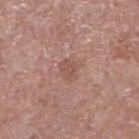{
  "biopsy_status": "not biopsied; imaged during a skin examination",
  "image": {
    "source": "total-body photography crop",
    "field_of_view_mm": 15
  },
  "site": "right forearm",
  "lighting": "white-light",
  "patient": {
    "sex": "male",
    "age_approx": 55
  },
  "lesion_size": {
    "long_diameter_mm_approx": 2.5
  },
  "automated_metrics": {
    "border_irregularity_0_10": 3.0,
    "color_variation_0_10": 2.0,
    "lesion_detection_confidence_0_100": 100
  }
}